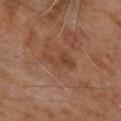Measured at roughly 4 mm in maximum diameter. An algorithmic analysis of the crop reported an area of roughly 6 mm², an outline eccentricity of about 0.9 (0 = round, 1 = elongated), and a symmetry-axis asymmetry near 0.45. It also reported a classifier nevus-likeness of about 0/100 and lesion-presence confidence of about 100/100. A 15 mm crop from a total-body photograph taken for skin-cancer surveillance. The tile uses cross-polarized illumination. Located on the upper back. A male subject, in their 70s.